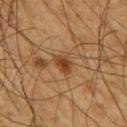{
  "biopsy_status": "not biopsied; imaged during a skin examination",
  "image": {
    "source": "total-body photography crop",
    "field_of_view_mm": 15
  },
  "patient": {
    "sex": "male",
    "age_approx": 60
  },
  "lighting": "cross-polarized",
  "automated_metrics": {
    "area_mm2_approx": 4.0,
    "eccentricity": 0.6,
    "shape_asymmetry": 0.3
  },
  "site": "arm"
}